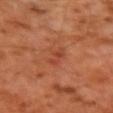Impression: Part of a total-body skin-imaging series; this lesion was reviewed on a skin check and was not flagged for biopsy. Acquisition and patient details: This image is a 15 mm lesion crop taken from a total-body photograph. The lesion's longest dimension is about 3 mm. Captured under cross-polarized illumination. The lesion is on the left upper arm. A male patient aged around 60.